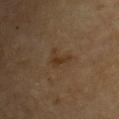Assessment:
Part of a total-body skin-imaging series; this lesion was reviewed on a skin check and was not flagged for biopsy.
Context:
On the chest. Approximately 2.5 mm at its widest. A male patient aged approximately 85 or older. A lesion tile, about 15 mm wide, cut from a 3D total-body photograph. The tile uses cross-polarized illumination.